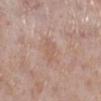This lesion was catalogued during total-body skin photography and was not selected for biopsy.
The total-body-photography lesion software estimated an area of roughly 4.5 mm², a shape eccentricity near 0.85, and a shape-asymmetry score of about 0.4 (0 = symmetric).
Captured under white-light illumination.
On the left lower leg.
A female patient approximately 60 years of age.
Measured at roughly 3.5 mm in maximum diameter.
This image is a 15 mm lesion crop taken from a total-body photograph.